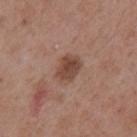{
  "biopsy_status": "not biopsied; imaged during a skin examination",
  "lighting": "white-light",
  "image": {
    "source": "total-body photography crop",
    "field_of_view_mm": 15
  },
  "patient": {
    "sex": "male",
    "age_approx": 55
  },
  "site": "mid back",
  "lesion_size": {
    "long_diameter_mm_approx": 3.0
  },
  "automated_metrics": {
    "area_mm2_approx": 6.5,
    "eccentricity": 0.65,
    "shape_asymmetry": 0.2
  }
}